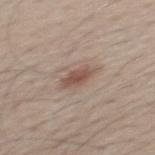{"biopsy_status": "not biopsied; imaged during a skin examination", "lighting": "white-light", "image": {"source": "total-body photography crop", "field_of_view_mm": 15}, "patient": {"sex": "male", "age_approx": 40}, "lesion_size": {"long_diameter_mm_approx": 3.0}, "site": "mid back"}